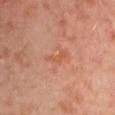Imaged during a routine full-body skin examination; the lesion was not biopsied and no histopathology is available. The lesion is on the left upper arm. Captured under cross-polarized illumination. Approximately 3 mm at its widest. This image is a 15 mm lesion crop taken from a total-body photograph. A male subject, aged 28 to 32.